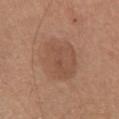| key | value |
|---|---|
| patient | male, aged around 65 |
| acquisition | total-body-photography crop, ~15 mm field of view |
| location | the chest |